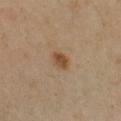location = the left forearm; lighting = cross-polarized illumination; automated metrics = a lesion area of about 4 mm² and a symmetry-axis asymmetry near 0.2; subject = male, aged approximately 50; image = total-body-photography crop, ~15 mm field of view.